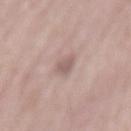The lesion was tiled from a total-body skin photograph and was not biopsied.